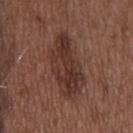Q: Is there a histopathology result?
A: imaged on a skin check; not biopsied
Q: How large is the lesion?
A: ~8 mm (longest diameter)
Q: Patient demographics?
A: male, approximately 50 years of age
Q: What did automated image analysis measure?
A: a footprint of about 23 mm² and a shape-asymmetry score of about 0.25 (0 = symmetric); an average lesion color of about L≈34 a*≈19 b*≈23 (CIELAB), a lesion–skin lightness drop of about 9, and a normalized border contrast of about 8
Q: How was this image acquired?
A: total-body-photography crop, ~15 mm field of view
Q: What is the anatomic site?
A: the head or neck